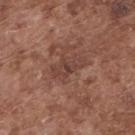Recorded during total-body skin imaging; not selected for excision or biopsy.
Located on the upper back.
The tile uses white-light illumination.
The patient is a male about 75 years old.
The recorded lesion diameter is about 4 mm.
Cropped from a whole-body photographic skin survey; the tile spans about 15 mm.